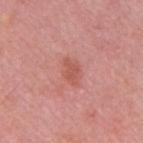Part of a total-body skin-imaging series; this lesion was reviewed on a skin check and was not flagged for biopsy. A male patient aged approximately 50. Located on the mid back. A lesion tile, about 15 mm wide, cut from a 3D total-body photograph.